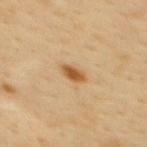The lesion was tiled from a total-body skin photograph and was not biopsied. Located on the upper back. A 15 mm close-up tile from a total-body photography series done for melanoma screening. Captured under cross-polarized illumination. A female subject aged approximately 50. Approximately 2.5 mm at its widest. Automated image analysis of the tile measured a footprint of about 3.5 mm², an eccentricity of roughly 0.75, and a symmetry-axis asymmetry near 0.3. The analysis additionally found a mean CIELAB color near L≈55 a*≈21 b*≈41, roughly 13 lightness units darker than nearby skin, and a normalized lesion–skin contrast near 9.5. And it measured border irregularity of about 2.5 on a 0–10 scale and a peripheral color-asymmetry measure near 0.5.